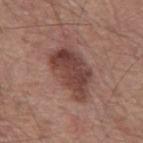<tbp_lesion>
  <lighting>white-light</lighting>
  <site>mid back</site>
  <patient>
    <sex>male</sex>
    <age_approx>55</age_approx>
  </patient>
  <lesion_size>
    <long_diameter_mm_approx>6.5</long_diameter_mm_approx>
  </lesion_size>
  <image>
    <source>total-body photography crop</source>
    <field_of_view_mm>15</field_of_view_mm>
  </image>
  <automated_metrics>
    <border_irregularity_0_10>3.5</border_irregularity_0_10>
    <color_variation_0_10>4.5</color_variation_0_10>
    <peripheral_color_asymmetry>1.5</peripheral_color_asymmetry>
    <nevus_likeness_0_100>65</nevus_likeness_0_100>
    <lesion_detection_confidence_0_100>100</lesion_detection_confidence_0_100>
  </automated_metrics>
</tbp_lesion>